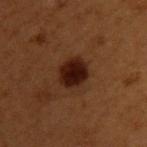Findings:
* follow-up · total-body-photography surveillance lesion; no biopsy
* automated lesion analysis · a nevus-likeness score of about 95/100 and lesion-presence confidence of about 100/100
* lighting · cross-polarized
* lesion size · ≈3.5 mm
* anatomic site · the upper back
* patient · male, about 50 years old
* image · total-body-photography crop, ~15 mm field of view